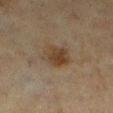Assessment: Recorded during total-body skin imaging; not selected for excision or biopsy. Clinical summary: The subject is a female in their mid-50s. From the left lower leg. This image is a 15 mm lesion crop taken from a total-body photograph.